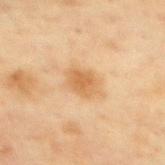Imaged during a routine full-body skin examination; the lesion was not biopsied and no histopathology is available.